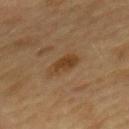{
  "biopsy_status": "not biopsied; imaged during a skin examination",
  "patient": {
    "sex": "female",
    "age_approx": 60
  },
  "site": "mid back",
  "image": {
    "source": "total-body photography crop",
    "field_of_view_mm": 15
  }
}